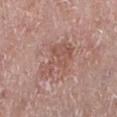Assessment: This lesion was catalogued during total-body skin photography and was not selected for biopsy. Background: A male patient, in their mid- to late 70s. Longest diameter approximately 5 mm. The lesion is located on the left lower leg. A region of skin cropped from a whole-body photographic capture, roughly 15 mm wide.